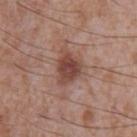{"biopsy_status": "not biopsied; imaged during a skin examination", "image": {"source": "total-body photography crop", "field_of_view_mm": 15}, "patient": {"sex": "male", "age_approx": 45}, "site": "front of the torso", "lighting": "white-light"}